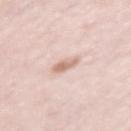Q: Was this lesion biopsied?
A: catalogued during a skin exam; not biopsied
Q: How was the tile lit?
A: white-light illumination
Q: What is the imaging modality?
A: ~15 mm tile from a whole-body skin photo
Q: Patient demographics?
A: female, in their mid- to late 60s
Q: What is the anatomic site?
A: the left upper arm
Q: Lesion size?
A: ~2.5 mm (longest diameter)